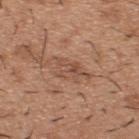Findings:
– automated metrics · a footprint of about 8.5 mm², an eccentricity of roughly 0.85, and a symmetry-axis asymmetry near 0.3; about 8 CIELAB-L* units darker than the surrounding skin
– subject · male, about 40 years old
– lesion diameter · ≈5 mm
– acquisition · 15 mm crop, total-body photography
– tile lighting · white-light
– body site · the upper back